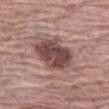No biopsy was performed on this lesion — it was imaged during a full skin examination and was not determined to be concerning. The subject is a male in their 70s. On the left thigh. A close-up tile cropped from a whole-body skin photograph, about 15 mm across. An algorithmic analysis of the crop reported a color-variation rating of about 4.5/10 and radial color variation of about 1.5. Imaged with white-light lighting. Longest diameter approximately 5 mm.